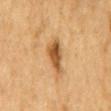Captured during whole-body skin photography for melanoma surveillance; the lesion was not biopsied.
On the mid back.
This image is a 15 mm lesion crop taken from a total-body photograph.
The patient is a male in their mid-80s or older.